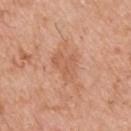Q: Was this lesion biopsied?
A: imaged on a skin check; not biopsied
Q: How was this image acquired?
A: total-body-photography crop, ~15 mm field of view
Q: Who is the patient?
A: male, aged 73–77
Q: Automated lesion metrics?
A: an area of roughly 7 mm² and a shape-asymmetry score of about 0.4 (0 = symmetric); a border-irregularity rating of about 4/10, internal color variation of about 2.5 on a 0–10 scale, and peripheral color asymmetry of about 1; an automated nevus-likeness rating near 0 out of 100 and lesion-presence confidence of about 100/100
Q: Illumination type?
A: white-light
Q: Lesion location?
A: the upper back